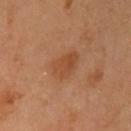workup: catalogued during a skin exam; not biopsied | patient: female, aged approximately 60 | location: the left upper arm | imaging modality: ~15 mm crop, total-body skin-cancer survey.